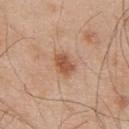follow-up: imaged on a skin check; not biopsied | subject: male, aged 53 to 57 | automated metrics: a lesion color around L≈54 a*≈23 b*≈33 in CIELAB, about 11 CIELAB-L* units darker than the surrounding skin, and a lesion-to-skin contrast of about 8 (normalized; higher = more distinct) | diameter: about 3 mm | site: the upper back | image source: total-body-photography crop, ~15 mm field of view.